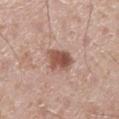Part of a total-body skin-imaging series; this lesion was reviewed on a skin check and was not flagged for biopsy.
A male patient approximately 35 years of age.
Measured at roughly 4 mm in maximum diameter.
A region of skin cropped from a whole-body photographic capture, roughly 15 mm wide.
The tile uses white-light illumination.
From the right lower leg.
The lesion-visualizer software estimated a footprint of about 8 mm², a shape eccentricity near 0.7, and a symmetry-axis asymmetry near 0.2. The analysis additionally found a border-irregularity index near 2/10 and peripheral color asymmetry of about 2.